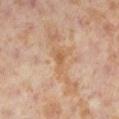Assessment: Captured during whole-body skin photography for melanoma surveillance; the lesion was not biopsied. Clinical summary: A 15 mm close-up extracted from a 3D total-body photography capture. A female patient, in their 40s. Located on the left lower leg.